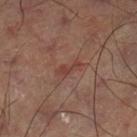biopsy_status: not biopsied; imaged during a skin examination
image:
  source: total-body photography crop
  field_of_view_mm: 15
lesion_size:
  long_diameter_mm_approx: 3.0
lighting: cross-polarized
site: left lower leg
automated_metrics:
  nevus_likeness_0_100: 0
  lesion_detection_confidence_0_100: 100